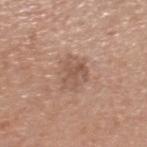Notes:
* follow-up · no biopsy performed (imaged during a skin exam)
* tile lighting · white-light illumination
* image · ~15 mm tile from a whole-body skin photo
* subject · male, aged 58–62
* image-analysis metrics · an average lesion color of about L≈55 a*≈19 b*≈27 (CIELAB), a lesion–skin lightness drop of about 8, and a lesion-to-skin contrast of about 6 (normalized; higher = more distinct); an automated nevus-likeness rating near 0 out of 100 and a lesion-detection confidence of about 100/100
* body site · the head or neck